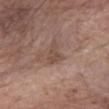Assessment: Part of a total-body skin-imaging series; this lesion was reviewed on a skin check and was not flagged for biopsy. Acquisition and patient details: A male patient, aged around 60. The lesion-visualizer software estimated an eccentricity of roughly 0.85 and a shape-asymmetry score of about 0.4 (0 = symmetric). The analysis additionally found a lesion color around L≈48 a*≈17 b*≈25 in CIELAB and a lesion-to-skin contrast of about 6 (normalized; higher = more distinct). On the left forearm. Captured under white-light illumination. About 3.5 mm across. A lesion tile, about 15 mm wide, cut from a 3D total-body photograph.